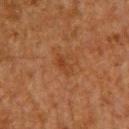biopsy_status: not biopsied; imaged during a skin examination
patient:
  sex: male
  age_approx: 60
image:
  source: total-body photography crop
  field_of_view_mm: 15
automated_metrics:
  border_irregularity_0_10: 3.0
  color_variation_0_10: 3.5
  peripheral_color_asymmetry: 1.0
site: upper back
lesion_size:
  long_diameter_mm_approx: 3.0
lighting: cross-polarized